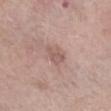No biopsy was performed on this lesion — it was imaged during a full skin examination and was not determined to be concerning. A 15 mm close-up tile from a total-body photography series done for melanoma screening. Automated image analysis of the tile measured an area of roughly 4 mm², an eccentricity of roughly 0.6, and two-axis asymmetry of about 0.3. And it measured a lesion color around L≈56 a*≈19 b*≈23 in CIELAB and a normalized lesion–skin contrast near 5.5. It also reported border irregularity of about 3.5 on a 0–10 scale and a color-variation rating of about 1.5/10. Imaged with white-light lighting. The lesion is on the left forearm. A male subject aged approximately 60. About 2.5 mm across.